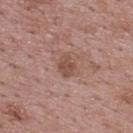notes = catalogued during a skin exam; not biopsied | anatomic site = the upper back | lesion size = ~2.5 mm (longest diameter) | tile lighting = white-light illumination | subject = male, in their 70s | imaging modality = ~15 mm crop, total-body skin-cancer survey | TBP lesion metrics = a shape eccentricity near 0.65 and a symmetry-axis asymmetry near 0.2.